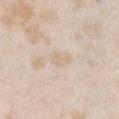{
  "biopsy_status": "not biopsied; imaged during a skin examination"
}